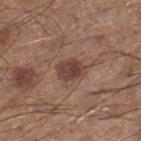Recorded during total-body skin imaging; not selected for excision or biopsy. The lesion's longest dimension is about 3.5 mm. Automated tile analysis of the lesion measured a lesion area of about 6.5 mm², an outline eccentricity of about 0.65 (0 = round, 1 = elongated), and a shape-asymmetry score of about 0.15 (0 = symmetric). The analysis additionally found a mean CIELAB color near L≈42 a*≈19 b*≈23, about 11 CIELAB-L* units darker than the surrounding skin, and a lesion-to-skin contrast of about 8.5 (normalized; higher = more distinct). The analysis additionally found border irregularity of about 1.5 on a 0–10 scale. It also reported a nevus-likeness score of about 75/100 and lesion-presence confidence of about 100/100. A 15 mm crop from a total-body photograph taken for skin-cancer surveillance. This is a white-light tile. From the right lower leg. The patient is a male about 60 years old.